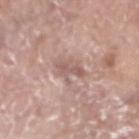Assessment:
Recorded during total-body skin imaging; not selected for excision or biopsy.
Clinical summary:
This is a white-light tile. A male patient, aged approximately 65. About 4 mm across. From the head or neck. A roughly 15 mm field-of-view crop from a total-body skin photograph.